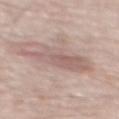Captured during whole-body skin photography for melanoma surveillance; the lesion was not biopsied.
The subject is a male roughly 70 years of age.
This is a white-light tile.
Measured at roughly 6.5 mm in maximum diameter.
A lesion tile, about 15 mm wide, cut from a 3D total-body photograph.
The lesion-visualizer software estimated an area of roughly 14 mm² and a shape-asymmetry score of about 0.4 (0 = symmetric). It also reported a lesion color around L≈59 a*≈18 b*≈21 in CIELAB, roughly 9 lightness units darker than nearby skin, and a normalized lesion–skin contrast near 6. And it measured internal color variation of about 3.5 on a 0–10 scale and peripheral color asymmetry of about 1. And it measured a classifier nevus-likeness of about 0/100.
On the mid back.